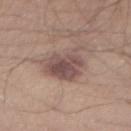The lesion was tiled from a total-body skin photograph and was not biopsied.